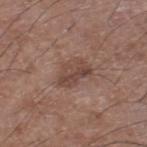Image and clinical context: A male patient aged around 60. The lesion is located on the right lower leg. Longest diameter approximately 4 mm. The tile uses white-light illumination. Cropped from a total-body skin-imaging series; the visible field is about 15 mm.